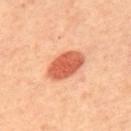Case summary:
• patient: female, about 45 years old
• body site: the upper back
• image: ~15 mm tile from a whole-body skin photo
• tile lighting: cross-polarized illumination
• lesion size: ~5 mm (longest diameter)